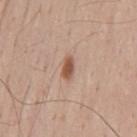This lesion was catalogued during total-body skin photography and was not selected for biopsy.
On the mid back.
About 2.5 mm across.
Captured under white-light illumination.
A 15 mm close-up extracted from a 3D total-body photography capture.
A male subject, in their mid-30s.